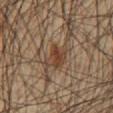Imaged during a routine full-body skin examination; the lesion was not biopsied and no histopathology is available. Automated tile analysis of the lesion measured a shape eccentricity near 0.7. It also reported border irregularity of about 3.5 on a 0–10 scale, a within-lesion color-variation index near 5/10, and radial color variation of about 1.5. From the abdomen. This is a cross-polarized tile. A 15 mm crop from a total-body photograph taken for skin-cancer surveillance. The subject is a male approximately 60 years of age. Approximately 3.5 mm at its widest.